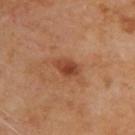workup=total-body-photography surveillance lesion; no biopsy
tile lighting=cross-polarized illumination
image-analysis metrics=border irregularity of about 2.5 on a 0–10 scale, a within-lesion color-variation index near 5/10, and a peripheral color-asymmetry measure near 2
patient=male, about 70 years old
anatomic site=the upper back
diameter=about 3 mm
image=total-body-photography crop, ~15 mm field of view